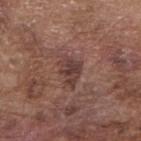Background: From the right forearm. The recorded lesion diameter is about 3.5 mm. Cropped from a whole-body photographic skin survey; the tile spans about 15 mm. A male patient aged approximately 75.